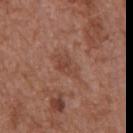Imaged during a routine full-body skin examination; the lesion was not biopsied and no histopathology is available. A male subject aged approximately 75. This is a white-light tile. Measured at roughly 3.5 mm in maximum diameter. From the chest. This image is a 15 mm lesion crop taken from a total-body photograph.